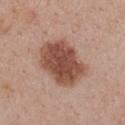Located on the chest.
The lesion's longest dimension is about 6.5 mm.
The patient is a female approximately 55 years of age.
This image is a 15 mm lesion crop taken from a total-body photograph.
Imaged with white-light lighting.
The lesion-visualizer software estimated border irregularity of about 1.5 on a 0–10 scale, internal color variation of about 4 on a 0–10 scale, and radial color variation of about 1.